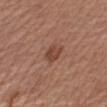Recorded during total-body skin imaging; not selected for excision or biopsy.
The lesion is located on the right upper arm.
Longest diameter approximately 3 mm.
Captured under white-light illumination.
A male patient approximately 75 years of age.
A 15 mm close-up tile from a total-body photography series done for melanoma screening.